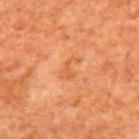Clinical impression: No biopsy was performed on this lesion — it was imaged during a full skin examination and was not determined to be concerning. Image and clinical context: On the back. A 15 mm close-up tile from a total-body photography series done for melanoma screening. A male patient roughly 80 years of age. Automated image analysis of the tile measured a mean CIELAB color near L≈47 a*≈23 b*≈36, about 5 CIELAB-L* units darker than the surrounding skin, and a lesion-to-skin contrast of about 5 (normalized; higher = more distinct). The analysis additionally found a border-irregularity rating of about 6/10 and a color-variation rating of about 1/10. Longest diameter approximately 3 mm. Captured under cross-polarized illumination.